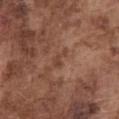The lesion is on the front of the torso. A lesion tile, about 15 mm wide, cut from a 3D total-body photograph. Imaged with white-light lighting. The subject is a male roughly 75 years of age. The total-body-photography lesion software estimated a footprint of about 2.5 mm², an outline eccentricity of about 0.85 (0 = round, 1 = elongated), and a shape-asymmetry score of about 0.6 (0 = symmetric). It also reported an average lesion color of about L≈42 a*≈21 b*≈28 (CIELAB), about 7 CIELAB-L* units darker than the surrounding skin, and a normalized border contrast of about 5.5. It also reported a within-lesion color-variation index near 1/10 and radial color variation of about 0.5. The software also gave an automated nevus-likeness rating near 0 out of 100 and a lesion-detection confidence of about 100/100. About 2.5 mm across.